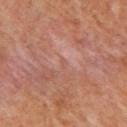Case summary:
* workup: imaged on a skin check; not biopsied
* subject: female, aged approximately 50
* lighting: cross-polarized illumination
* acquisition: 15 mm crop, total-body photography
* automated lesion analysis: a lesion area of about 1 mm², an eccentricity of roughly 0.9, and a shape-asymmetry score of about 0.6 (0 = symmetric)
* anatomic site: the upper back
* lesion size: about 1.5 mm